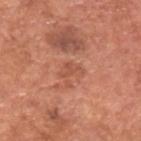* notes: no biopsy performed (imaged during a skin exam)
* diameter: about 3 mm
* lighting: white-light
* patient: male, in their mid- to late 60s
* imaging modality: ~15 mm crop, total-body skin-cancer survey
* anatomic site: the upper back